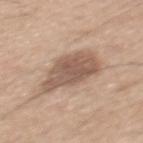The lesion was tiled from a total-body skin photograph and was not biopsied. Cropped from a total-body skin-imaging series; the visible field is about 15 mm. A male subject, about 45 years old. The lesion is on the mid back.